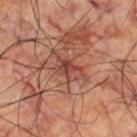Imaged during a routine full-body skin examination; the lesion was not biopsied and no histopathology is available.
The lesion is on the right thigh.
A male patient about 60 years old.
The recorded lesion diameter is about 4 mm.
A region of skin cropped from a whole-body photographic capture, roughly 15 mm wide.
The total-body-photography lesion software estimated a footprint of about 6 mm² and a symmetry-axis asymmetry near 0.35. The analysis additionally found about 9 CIELAB-L* units darker than the surrounding skin and a normalized lesion–skin contrast near 7. It also reported a border-irregularity rating of about 5/10, a color-variation rating of about 6/10, and radial color variation of about 2.5.